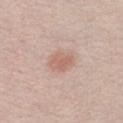Impression: No biopsy was performed on this lesion — it was imaged during a full skin examination and was not determined to be concerning. Context: Imaged with white-light lighting. About 3 mm across. A 15 mm close-up extracted from a 3D total-body photography capture. The patient is a male aged 28 to 32.